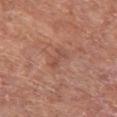Imaged during a routine full-body skin examination; the lesion was not biopsied and no histopathology is available.
A male patient roughly 65 years of age.
This image is a 15 mm lesion crop taken from a total-body photograph.
Automated tile analysis of the lesion measured an area of roughly 2.5 mm², a shape eccentricity near 0.9, and two-axis asymmetry of about 0.35. The analysis additionally found border irregularity of about 5 on a 0–10 scale, internal color variation of about 0 on a 0–10 scale, and peripheral color asymmetry of about 0.
Approximately 3 mm at its widest.
The lesion is on the left thigh.